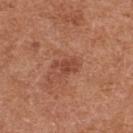This lesion was catalogued during total-body skin photography and was not selected for biopsy. The lesion is on the back. Imaged with white-light lighting. The lesion's longest dimension is about 3 mm. This image is a 15 mm lesion crop taken from a total-body photograph. The subject is a female in their 40s.